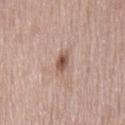The lesion was photographed on a routine skin check and not biopsied; there is no pathology result. A 15 mm close-up tile from a total-body photography series done for melanoma screening. Longest diameter approximately 2.5 mm. The lesion is on the mid back. A male subject aged approximately 55.